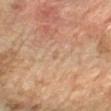{
  "biopsy_status": "not biopsied; imaged during a skin examination",
  "image": {
    "source": "total-body photography crop",
    "field_of_view_mm": 15
  },
  "patient": {
    "sex": "female",
    "age_approx": 60
  },
  "site": "left forearm"
}